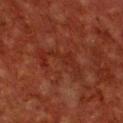Acquisition and patient details: A male subject aged around 60. Cropped from a total-body skin-imaging series; the visible field is about 15 mm. On the chest.